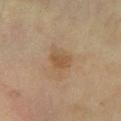Acquisition and patient details:
A close-up tile cropped from a whole-body skin photograph, about 15 mm across. The tile uses cross-polarized illumination. From the left lower leg. A male patient, roughly 65 years of age. Longest diameter approximately 3 mm.